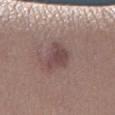<record>
  <biopsy_status>not biopsied; imaged during a skin examination</biopsy_status>
  <site>right lower leg</site>
  <lesion_size>
    <long_diameter_mm_approx>4.0</long_diameter_mm_approx>
  </lesion_size>
  <image>
    <source>total-body photography crop</source>
    <field_of_view_mm>15</field_of_view_mm>
  </image>
  <automated_metrics>
    <cielab_L>45</cielab_L>
    <cielab_a>18</cielab_a>
    <cielab_b>18</cielab_b>
    <vs_skin_darker_L>9.0</vs_skin_darker_L>
    <vs_skin_contrast_norm>7.5</vs_skin_contrast_norm>
    <border_irregularity_0_10>4.0</border_irregularity_0_10>
    <color_variation_0_10>2.5</color_variation_0_10>
    <peripheral_color_asymmetry>1.0</peripheral_color_asymmetry>
    <nevus_likeness_0_100>10</nevus_likeness_0_100>
  </automated_metrics>
  <lighting>white-light</lighting>
  <patient>
    <sex>female</sex>
    <age_approx>30</age_approx>
  </patient>
</record>